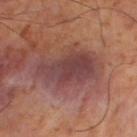Notes:
• biopsy status — total-body-photography surveillance lesion; no biopsy
• body site — the right lower leg
• automated lesion analysis — a nevus-likeness score of about 0/100
• patient — male, aged 53–57
• lesion diameter — ≈7 mm
• image source — total-body-photography crop, ~15 mm field of view